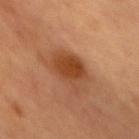Impression: Captured during whole-body skin photography for melanoma surveillance; the lesion was not biopsied. Context: The patient is a female roughly 60 years of age. Approximately 5 mm at its widest. A region of skin cropped from a whole-body photographic capture, roughly 15 mm wide. This is a cross-polarized tile. The total-body-photography lesion software estimated an eccentricity of roughly 0.6 and a symmetry-axis asymmetry near 0.25. The software also gave a lesion color around L≈39 a*≈22 b*≈33 in CIELAB, about 9 CIELAB-L* units darker than the surrounding skin, and a normalized border contrast of about 8.5. And it measured border irregularity of about 3 on a 0–10 scale, internal color variation of about 3 on a 0–10 scale, and peripheral color asymmetry of about 1. The lesion is on the front of the torso.